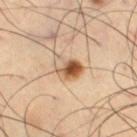| field | value |
|---|---|
| notes | total-body-photography surveillance lesion; no biopsy |
| patient | male, aged 63–67 |
| lighting | cross-polarized illumination |
| lesion diameter | about 3.5 mm |
| site | the right thigh |
| imaging modality | ~15 mm tile from a whole-body skin photo |